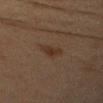Captured during whole-body skin photography for melanoma surveillance; the lesion was not biopsied. A male subject roughly 45 years of age. A region of skin cropped from a whole-body photographic capture, roughly 15 mm wide. The lesion's longest dimension is about 3.5 mm. Automated tile analysis of the lesion measured an area of roughly 4 mm², a shape eccentricity near 0.9, and a shape-asymmetry score of about 0.35 (0 = symmetric). It also reported a nevus-likeness score of about 25/100. The tile uses cross-polarized illumination. Located on the arm.